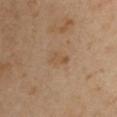{"biopsy_status": "not biopsied; imaged during a skin examination", "automated_metrics": {"cielab_L": 50, "cielab_a": 16, "cielab_b": 32, "vs_skin_darker_L": 5.0, "vs_skin_contrast_norm": 5.0}, "site": "right upper arm", "lighting": "cross-polarized", "lesion_size": {"long_diameter_mm_approx": 3.0}, "image": {"source": "total-body photography crop", "field_of_view_mm": 15}, "patient": {"sex": "male", "age_approx": 40}}